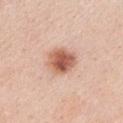Recorded during total-body skin imaging; not selected for excision or biopsy. Measured at roughly 3.5 mm in maximum diameter. A region of skin cropped from a whole-body photographic capture, roughly 15 mm wide. The patient is a female about 45 years old. Located on the chest.